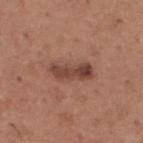Part of a total-body skin-imaging series; this lesion was reviewed on a skin check and was not flagged for biopsy. A lesion tile, about 15 mm wide, cut from a 3D total-body photograph. Automated tile analysis of the lesion measured a footprint of about 8 mm², a shape eccentricity near 0.9, and a symmetry-axis asymmetry near 0.25. And it measured a border-irregularity rating of about 3/10, internal color variation of about 4.5 on a 0–10 scale, and radial color variation of about 1.5. A male patient, approximately 65 years of age. From the upper back. Longest diameter approximately 5 mm. This is a white-light tile.